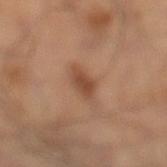The lesion was tiled from a total-body skin photograph and was not biopsied. Approximately 3 mm at its widest. The lesion is on the left leg. The subject is a male about 50 years old. A 15 mm close-up tile from a total-body photography series done for melanoma screening.